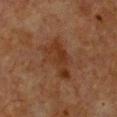biopsy status: imaged on a skin check; not biopsied
automated metrics: a lesion area of about 14 mm², an outline eccentricity of about 0.8 (0 = round, 1 = elongated), and two-axis asymmetry of about 0.4; a border-irregularity index near 4.5/10, a color-variation rating of about 3/10, and peripheral color asymmetry of about 1; a detector confidence of about 100 out of 100 that the crop contains a lesion
patient: female, about 55 years old
diameter: ~5.5 mm (longest diameter)
image source: total-body-photography crop, ~15 mm field of view
location: the chest